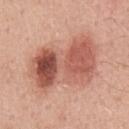Clinical impression: Captured during whole-body skin photography for melanoma surveillance; the lesion was not biopsied. Image and clinical context: The recorded lesion diameter is about 9 mm. Imaged with white-light lighting. The total-body-photography lesion software estimated a detector confidence of about 100 out of 100 that the crop contains a lesion. A male patient about 50 years old. On the chest. A close-up tile cropped from a whole-body skin photograph, about 15 mm across.